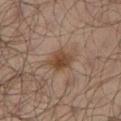| field | value |
|---|---|
| subject | male, aged 63 to 67 |
| location | the left lower leg |
| image | total-body-photography crop, ~15 mm field of view |